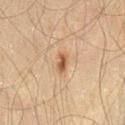Impression: Part of a total-body skin-imaging series; this lesion was reviewed on a skin check and was not flagged for biopsy. Background: An algorithmic analysis of the crop reported a lesion color around L≈44 a*≈15 b*≈28 in CIELAB. It also reported border irregularity of about 2 on a 0–10 scale, a color-variation rating of about 2.5/10, and a peripheral color-asymmetry measure near 0.5. It also reported a classifier nevus-likeness of about 90/100 and a detector confidence of about 100 out of 100 that the crop contains a lesion. Cropped from a whole-body photographic skin survey; the tile spans about 15 mm. The tile uses cross-polarized illumination. A male patient aged 58–62. From the lower back.